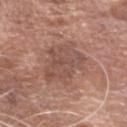Notes:
* biopsy status: catalogued during a skin exam; not biopsied
* acquisition: ~15 mm crop, total-body skin-cancer survey
* location: the head or neck
* automated lesion analysis: an area of roughly 17 mm² and a symmetry-axis asymmetry near 0.25; a nevus-likeness score of about 0/100
* lesion diameter: about 6.5 mm
* tile lighting: white-light illumination
* patient: male, in their mid- to late 70s